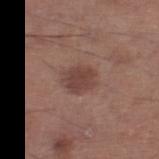biopsy status — imaged on a skin check; not biopsied
lighting — white-light
image — ~15 mm crop, total-body skin-cancer survey
location — the left lower leg
patient — male, approximately 65 years of age
diameter — ≈3.5 mm
image-analysis metrics — an area of roughly 7 mm² and an outline eccentricity of about 0.65 (0 = round, 1 = elongated)